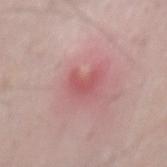workup = no biopsy performed (imaged during a skin exam) | body site = the mid back | image source = total-body-photography crop, ~15 mm field of view | subject = male, about 50 years old | tile lighting = white-light | lesion diameter = ~2.5 mm (longest diameter) | TBP lesion metrics = a mean CIELAB color near L≈54 a*≈31 b*≈22, about 8 CIELAB-L* units darker than the surrounding skin, and a normalized lesion–skin contrast near 5.5; a border-irregularity rating of about 5/10, a within-lesion color-variation index near 1/10, and radial color variation of about 0.5.